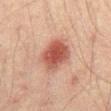{
  "biopsy_status": "not biopsied; imaged during a skin examination",
  "lesion_size": {
    "long_diameter_mm_approx": 4.5
  },
  "image": {
    "source": "total-body photography crop",
    "field_of_view_mm": 15
  },
  "lighting": "cross-polarized",
  "site": "abdomen",
  "patient": {
    "sex": "male",
    "age_approx": 45
  }
}